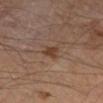Assessment: Part of a total-body skin-imaging series; this lesion was reviewed on a skin check and was not flagged for biopsy. Image and clinical context: A male patient in their mid-60s. This is a cross-polarized tile. The total-body-photography lesion software estimated a classifier nevus-likeness of about 60/100 and a detector confidence of about 100 out of 100 that the crop contains a lesion. Located on the right lower leg. The recorded lesion diameter is about 2.5 mm. Cropped from a total-body skin-imaging series; the visible field is about 15 mm.